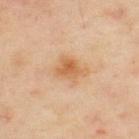Findings:
- follow-up — catalogued during a skin exam; not biopsied
- subject — male, roughly 60 years of age
- lesion size — about 3.5 mm
- acquisition — ~15 mm crop, total-body skin-cancer survey
- illumination — cross-polarized
- body site — the upper back